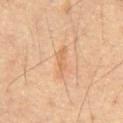* workup — no biopsy performed (imaged during a skin exam)
* patient — male, approximately 60 years of age
* image — ~15 mm crop, total-body skin-cancer survey
* diameter — ≈4 mm
* illumination — cross-polarized